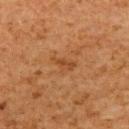Q: What is the imaging modality?
A: total-body-photography crop, ~15 mm field of view
Q: Patient demographics?
A: male, roughly 60 years of age
Q: What is the lesion's diameter?
A: ~3 mm (longest diameter)
Q: What is the anatomic site?
A: the arm